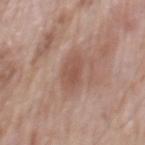Imaged during a routine full-body skin examination; the lesion was not biopsied and no histopathology is available.
On the mid back.
A 15 mm crop from a total-body photograph taken for skin-cancer surveillance.
A male patient, aged around 70.
The tile uses white-light illumination.
The lesion's longest dimension is about 3.5 mm.